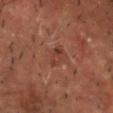Notes:
* follow-up · total-body-photography surveillance lesion; no biopsy
* lesion size · ~2.5 mm (longest diameter)
* TBP lesion metrics · an automated nevus-likeness rating near 5 out of 100 and lesion-presence confidence of about 100/100
* anatomic site · the head or neck
* lighting · cross-polarized illumination
* acquisition · 15 mm crop, total-body photography
* subject · male, aged 48–52